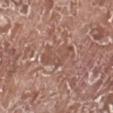{
  "biopsy_status": "not biopsied; imaged during a skin examination",
  "patient": {
    "sex": "male",
    "age_approx": 75
  },
  "lighting": "white-light",
  "automated_metrics": {
    "area_mm2_approx": 7.5,
    "eccentricity": 0.9,
    "shape_asymmetry": 0.5,
    "border_irregularity_0_10": 7.5,
    "nevus_likeness_0_100": 0
  },
  "site": "left lower leg",
  "image": {
    "source": "total-body photography crop",
    "field_of_view_mm": 15
  }
}